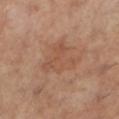notes = no biopsy performed (imaged during a skin exam)
illumination = cross-polarized
patient = female, in their 60s
lesion size = ≈5.5 mm
location = the left lower leg
image = 15 mm crop, total-body photography